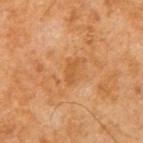Findings:
* biopsy status — no biopsy performed (imaged during a skin exam)
* image source — ~15 mm tile from a whole-body skin photo
* location — the right upper arm
* patient — aged around 65
* illumination — cross-polarized